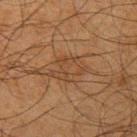No biopsy was performed on this lesion — it was imaged during a full skin examination and was not determined to be concerning.